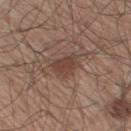Clinical impression:
Captured during whole-body skin photography for melanoma surveillance; the lesion was not biopsied.
Image and clinical context:
Automated tile analysis of the lesion measured a detector confidence of about 100 out of 100 that the crop contains a lesion. The lesion is on the leg. A roughly 15 mm field-of-view crop from a total-body skin photograph. A male patient, aged around 60. About 3 mm across.